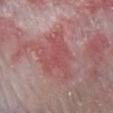workup — no biopsy performed (imaged during a skin exam).